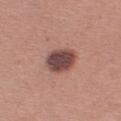biopsy status — total-body-photography surveillance lesion; no biopsy
anatomic site — the right upper arm
image — ~15 mm tile from a whole-body skin photo
automated metrics — a border-irregularity rating of about 1.5/10, a color-variation rating of about 3.5/10, and a peripheral color-asymmetry measure near 1; a classifier nevus-likeness of about 60/100 and lesion-presence confidence of about 100/100
subject — female, approximately 40 years of age